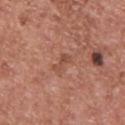Case summary:
* follow-up — no biopsy performed (imaged during a skin exam)
* image — 15 mm crop, total-body photography
* patient — male, aged approximately 70
* diameter — ~2.5 mm (longest diameter)
* lighting — white-light
* location — the upper back